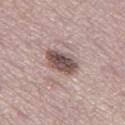Assessment:
This lesion was catalogued during total-body skin photography and was not selected for biopsy.
Acquisition and patient details:
A 15 mm crop from a total-body photograph taken for skin-cancer surveillance. On the left thigh. The subject is a female about 50 years old.